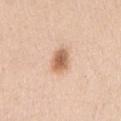The lesion was tiled from a total-body skin photograph and was not biopsied.
The recorded lesion diameter is about 3 mm.
A 15 mm close-up extracted from a 3D total-body photography capture.
The lesion is located on the right upper arm.
This is a white-light tile.
The subject is a female aged around 40.